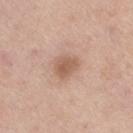biopsy status — total-body-photography surveillance lesion; no biopsy
image — ~15 mm tile from a whole-body skin photo
patient — male, about 75 years old
body site — the right thigh